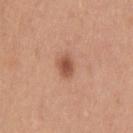<tbp_lesion>
  <biopsy_status>not biopsied; imaged during a skin examination</biopsy_status>
  <image>
    <source>total-body photography crop</source>
    <field_of_view_mm>15</field_of_view_mm>
  </image>
  <lesion_size>
    <long_diameter_mm_approx>2.5</long_diameter_mm_approx>
  </lesion_size>
  <lighting>white-light</lighting>
  <site>back</site>
  <patient>
    <sex>male</sex>
    <age_approx>40</age_approx>
  </patient>
  <automated_metrics>
    <eccentricity>0.6</eccentricity>
    <shape_asymmetry>0.2</shape_asymmetry>
    <vs_skin_darker_L>12.0</vs_skin_darker_L>
    <vs_skin_contrast_norm>8.0</vs_skin_contrast_norm>
    <color_variation_0_10>2.5</color_variation_0_10>
    <peripheral_color_asymmetry>0.5</peripheral_color_asymmetry>
    <nevus_likeness_0_100>90</nevus_likeness_0_100>
    <lesion_detection_confidence_0_100>100</lesion_detection_confidence_0_100>
  </automated_metrics>
</tbp_lesion>